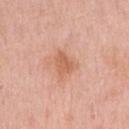Assessment: Recorded during total-body skin imaging; not selected for excision or biopsy. Image and clinical context: Measured at roughly 3 mm in maximum diameter. Cropped from a whole-body photographic skin survey; the tile spans about 15 mm. The lesion is located on the right upper arm. This is a white-light tile. Automated tile analysis of the lesion measured an outline eccentricity of about 0.45 (0 = round, 1 = elongated) and a symmetry-axis asymmetry near 0.45. The software also gave border irregularity of about 4 on a 0–10 scale and a within-lesion color-variation index near 2/10. The software also gave a nevus-likeness score of about 5/100 and a detector confidence of about 100 out of 100 that the crop contains a lesion. A female patient, aged 63 to 67.